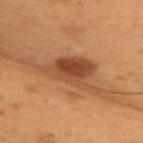Notes:
- follow-up — total-body-photography surveillance lesion; no biopsy
- lesion diameter — about 8 mm
- anatomic site — the upper back
- subject — male, aged around 55
- image source — 15 mm crop, total-body photography
- illumination — cross-polarized